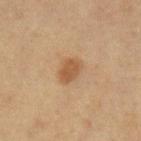Impression:
Captured during whole-body skin photography for melanoma surveillance; the lesion was not biopsied.
Image and clinical context:
The subject is a female aged 53 to 57. A lesion tile, about 15 mm wide, cut from a 3D total-body photograph. Located on the left lower leg.